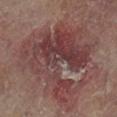This lesion was catalogued during total-body skin photography and was not selected for biopsy. An algorithmic analysis of the crop reported a lesion color around L≈35 a*≈19 b*≈17 in CIELAB, about 8 CIELAB-L* units darker than the surrounding skin, and a lesion-to-skin contrast of about 7.5 (normalized; higher = more distinct). It also reported border irregularity of about 8.5 on a 0–10 scale and a color-variation rating of about 7/10. It also reported lesion-presence confidence of about 85/100. The lesion is located on the left leg. This is a cross-polarized tile. Measured at roughly 10 mm in maximum diameter. A 15 mm close-up extracted from a 3D total-body photography capture. The patient is a female aged around 80.